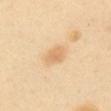biopsy_status: not biopsied; imaged during a skin examination
lighting: cross-polarized
patient:
  sex: female
  age_approx: 40
image:
  source: total-body photography crop
  field_of_view_mm: 15
lesion_size:
  long_diameter_mm_approx: 2.5
automated_metrics:
  area_mm2_approx: 4.0
  shape_asymmetry: 0.35
  border_irregularity_0_10: 3.0
  color_variation_0_10: 2.0
  peripheral_color_asymmetry: 0.5
  nevus_likeness_0_100: 90
  lesion_detection_confidence_0_100: 100
site: abdomen